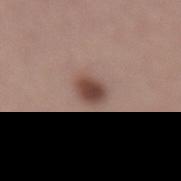notes — total-body-photography surveillance lesion; no biopsy